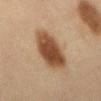notes = no biopsy performed (imaged during a skin exam); lighting = cross-polarized illumination; body site = the abdomen; lesion size = ~6.5 mm (longest diameter); subject = female, roughly 60 years of age; image source = total-body-photography crop, ~15 mm field of view.